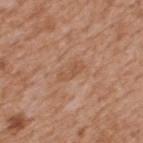Q: Is there a histopathology result?
A: total-body-photography surveillance lesion; no biopsy
Q: Lesion size?
A: ≈3 mm
Q: Patient demographics?
A: male, about 65 years old
Q: Where on the body is the lesion?
A: the upper back
Q: How was this image acquired?
A: total-body-photography crop, ~15 mm field of view